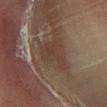The lesion was tiled from a total-body skin photograph and was not biopsied.
This is a cross-polarized tile.
This image is a 15 mm lesion crop taken from a total-body photograph.
From the left leg.
About 4.5 mm across.
The total-body-photography lesion software estimated a lesion color around L≈32 a*≈14 b*≈21 in CIELAB, a lesion–skin lightness drop of about 5, and a normalized border contrast of about 5. And it measured a within-lesion color-variation index near 1.5/10 and radial color variation of about 0.5.
A male patient aged around 55.